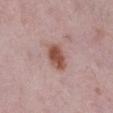Impression:
Part of a total-body skin-imaging series; this lesion was reviewed on a skin check and was not flagged for biopsy.
Context:
The lesion-visualizer software estimated a lesion area of about 7.5 mm², an eccentricity of roughly 0.75, and a shape-asymmetry score of about 0.15 (0 = symmetric). The analysis additionally found a mean CIELAB color near L≈52 a*≈23 b*≈25. The analysis additionally found an automated nevus-likeness rating near 95 out of 100 and a detector confidence of about 100 out of 100 that the crop contains a lesion. Imaged with white-light lighting. A female patient roughly 40 years of age. On the left lower leg. A 15 mm close-up tile from a total-body photography series done for melanoma screening.